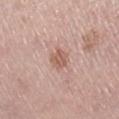Q: Was a biopsy performed?
A: imaged on a skin check; not biopsied